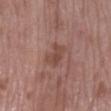Assessment:
Captured during whole-body skin photography for melanoma surveillance; the lesion was not biopsied.
Clinical summary:
Cropped from a total-body skin-imaging series; the visible field is about 15 mm. The total-body-photography lesion software estimated an eccentricity of roughly 0.8 and a shape-asymmetry score of about 0.25 (0 = symmetric). It also reported an average lesion color of about L≈46 a*≈21 b*≈25 (CIELAB) and roughly 8 lightness units darker than nearby skin. The software also gave a within-lesion color-variation index near 2/10. The analysis additionally found a classifier nevus-likeness of about 5/100 and a lesion-detection confidence of about 100/100. A female patient approximately 70 years of age. From the right lower leg.